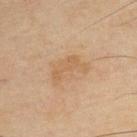{
  "biopsy_status": "not biopsied; imaged during a skin examination",
  "image": {
    "source": "total-body photography crop",
    "field_of_view_mm": 15
  },
  "patient": {
    "sex": "male",
    "age_approx": 60
  },
  "site": "upper back"
}